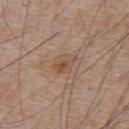| feature | finding |
|---|---|
| workup | no biopsy performed (imaged during a skin exam) |
| tile lighting | white-light illumination |
| lesion size | ~3 mm (longest diameter) |
| subject | male, aged 63 to 67 |
| TBP lesion metrics | a lesion color around L≈51 a*≈18 b*≈29 in CIELAB, a lesion–skin lightness drop of about 7, and a normalized border contrast of about 5.5; a border-irregularity rating of about 2/10, a color-variation rating of about 5/10, and radial color variation of about 1.5 |
| location | the upper back |
| image | 15 mm crop, total-body photography |